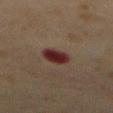Case summary:
* automated metrics · a shape eccentricity near 0.55 and a shape-asymmetry score of about 0.2 (0 = symmetric); a mean CIELAB color near L≈24 a*≈19 b*≈18 and a normalized lesion–skin contrast near 12.5; an automated nevus-likeness rating near 0 out of 100 and a lesion-detection confidence of about 100/100
* anatomic site · the abdomen
* illumination · cross-polarized
* image · total-body-photography crop, ~15 mm field of view
* patient · male, in their 60s